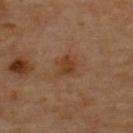Q: Was a biopsy performed?
A: catalogued during a skin exam; not biopsied
Q: Where on the body is the lesion?
A: the upper back
Q: How was the tile lit?
A: cross-polarized
Q: Patient demographics?
A: aged around 65
Q: What is the imaging modality?
A: ~15 mm crop, total-body skin-cancer survey
Q: Automated lesion metrics?
A: a footprint of about 5.5 mm² and two-axis asymmetry of about 0.3; a mean CIELAB color near L≈39 a*≈20 b*≈32 and a normalized lesion–skin contrast near 7; a border-irregularity rating of about 3/10, a color-variation rating of about 2/10, and peripheral color asymmetry of about 0.5; a classifier nevus-likeness of about 10/100 and lesion-presence confidence of about 100/100